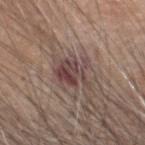Captured during whole-body skin photography for melanoma surveillance; the lesion was not biopsied. Automated tile analysis of the lesion measured a mean CIELAB color near L≈42 a*≈18 b*≈18 and about 10 CIELAB-L* units darker than the surrounding skin. And it measured border irregularity of about 6 on a 0–10 scale, a within-lesion color-variation index near 6.5/10, and peripheral color asymmetry of about 2.5. The lesion is on the head or neck. Imaged with white-light lighting. The lesion's longest dimension is about 3.5 mm. The patient is a male aged 58–62. A 15 mm crop from a total-body photograph taken for skin-cancer surveillance.